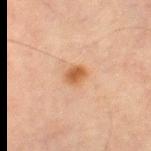Clinical impression: Recorded during total-body skin imaging; not selected for excision or biopsy. Context: The lesion-visualizer software estimated an area of roughly 4.5 mm², an eccentricity of roughly 0.7, and two-axis asymmetry of about 0.25. And it measured a border-irregularity index near 2.5/10 and internal color variation of about 3 on a 0–10 scale. The software also gave an automated nevus-likeness rating near 95 out of 100 and lesion-presence confidence of about 100/100. A male subject approximately 70 years of age. Located on the left thigh. A roughly 15 mm field-of-view crop from a total-body skin photograph.